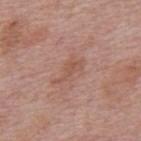{"automated_metrics": {"cielab_L": 53, "cielab_a": 22, "cielab_b": 27, "vs_skin_darker_L": 7.0, "vs_skin_contrast_norm": 5.5, "nevus_likeness_0_100": 0}, "lighting": "white-light", "patient": {"sex": "male", "age_approx": 75}, "image": {"source": "total-body photography crop", "field_of_view_mm": 15}, "site": "mid back"}